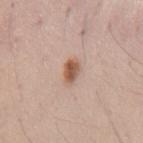| key | value |
|---|---|
| patient | male, aged 33–37 |
| image | 15 mm crop, total-body photography |
| site | the mid back |
| tile lighting | white-light |
| automated metrics | a lesion area of about 4.5 mm², an eccentricity of roughly 0.8, and a shape-asymmetry score of about 0.3 (0 = symmetric); an average lesion color of about L≈56 a*≈20 b*≈29 (CIELAB), roughly 13 lightness units darker than nearby skin, and a normalized border contrast of about 9.5; a border-irregularity rating of about 2.5/10, internal color variation of about 3.5 on a 0–10 scale, and a peripheral color-asymmetry measure near 1; an automated nevus-likeness rating near 95 out of 100 and a detector confidence of about 100 out of 100 that the crop contains a lesion |